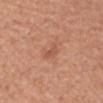<case>
<biopsy_status>not biopsied; imaged during a skin examination</biopsy_status>
<patient>
  <sex>female</sex>
  <age_approx>50</age_approx>
</patient>
<lighting>white-light</lighting>
<image>
  <source>total-body photography crop</source>
  <field_of_view_mm>15</field_of_view_mm>
</image>
<site>right forearm</site>
</case>